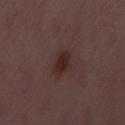Captured during whole-body skin photography for melanoma surveillance; the lesion was not biopsied. A lesion tile, about 15 mm wide, cut from a 3D total-body photograph. Imaged with white-light lighting. A male subject approximately 50 years of age. The lesion-visualizer software estimated a border-irregularity index near 2/10, internal color variation of about 2 on a 0–10 scale, and peripheral color asymmetry of about 0.5. And it measured an automated nevus-likeness rating near 85 out of 100 and lesion-presence confidence of about 100/100.